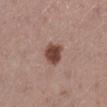Assessment:
Captured during whole-body skin photography for melanoma surveillance; the lesion was not biopsied.
Background:
The lesion is located on the left lower leg. Cropped from a total-body skin-imaging series; the visible field is about 15 mm. Captured under white-light illumination. A female subject roughly 50 years of age.